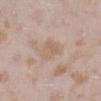Captured during whole-body skin photography for melanoma surveillance; the lesion was not biopsied. A female patient, roughly 25 years of age. On the left lower leg. A lesion tile, about 15 mm wide, cut from a 3D total-body photograph. Longest diameter approximately 3.5 mm.